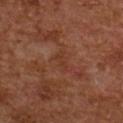notes=no biopsy performed (imaged during a skin exam)
acquisition=15 mm crop, total-body photography
size=about 2.5 mm
location=the upper back
patient=female, about 60 years old